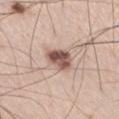This lesion was catalogued during total-body skin photography and was not selected for biopsy.
Imaged with white-light lighting.
A male subject, aged 53–57.
About 3 mm across.
This image is a 15 mm lesion crop taken from a total-body photograph.
The lesion-visualizer software estimated roughly 17 lightness units darker than nearby skin and a normalized border contrast of about 11. It also reported an automated nevus-likeness rating near 90 out of 100.
Located on the right thigh.